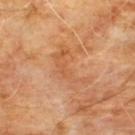Recorded during total-body skin imaging; not selected for excision or biopsy. A male subject approximately 60 years of age. On the front of the torso. A 15 mm close-up tile from a total-body photography series done for melanoma screening.